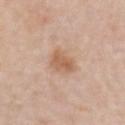This lesion was catalogued during total-body skin photography and was not selected for biopsy. The lesion is located on the left upper arm. Imaged with white-light lighting. A male patient aged 73–77. A lesion tile, about 15 mm wide, cut from a 3D total-body photograph. The recorded lesion diameter is about 3.5 mm.